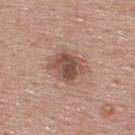  biopsy_status: not biopsied; imaged during a skin examination
  site: back
  image:
    source: total-body photography crop
    field_of_view_mm: 15
  patient:
    sex: male
    age_approx: 55